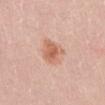Case summary:
• biopsy status · imaged on a skin check; not biopsied
• patient · female, roughly 15 years of age
• automated metrics · an average lesion color of about L≈63 a*≈23 b*≈32 (CIELAB), roughly 10 lightness units darker than nearby skin, and a normalized lesion–skin contrast near 7
• imaging modality · 15 mm crop, total-body photography
• site · the abdomen
• illumination · white-light
• lesion size · ~4 mm (longest diameter)